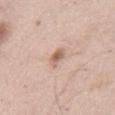Part of a total-body skin-imaging series; this lesion was reviewed on a skin check and was not flagged for biopsy.
The subject is a male aged approximately 55.
This image is a 15 mm lesion crop taken from a total-body photograph.
On the chest.